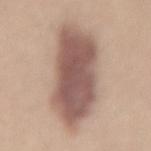Notes:
- biopsy status · catalogued during a skin exam; not biopsied
- tile lighting · white-light illumination
- image · ~15 mm crop, total-body skin-cancer survey
- subject · female, in their mid-30s
- automated metrics · a footprint of about 40 mm², an eccentricity of roughly 0.95, and two-axis asymmetry of about 0.15; a lesion color around L≈55 a*≈18 b*≈23 in CIELAB, about 16 CIELAB-L* units darker than the surrounding skin, and a normalized lesion–skin contrast near 10.5; a classifier nevus-likeness of about 80/100
- size · about 11.5 mm
- site · the mid back